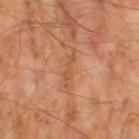Case summary:
* follow-up — catalogued during a skin exam; not biopsied
* image — 15 mm crop, total-body photography
* patient — male, aged approximately 65
* automated lesion analysis — a border-irregularity index near 6.5/10 and a peripheral color-asymmetry measure near 0; a classifier nevus-likeness of about 0/100 and a detector confidence of about 70 out of 100 that the crop contains a lesion
* size — ~4.5 mm (longest diameter)